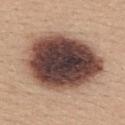<tbp_lesion>
<biopsy_status>not biopsied; imaged during a skin examination</biopsy_status>
<image>
  <source>total-body photography crop</source>
  <field_of_view_mm>15</field_of_view_mm>
</image>
<lighting>white-light</lighting>
<patient>
  <sex>female</sex>
  <age_approx>35</age_approx>
</patient>
<lesion_size>
  <long_diameter_mm_approx>10.0</long_diameter_mm_approx>
</lesion_size>
<site>upper back</site>
</tbp_lesion>